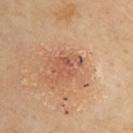Q: Is there a histopathology result?
A: no biopsy performed (imaged during a skin exam)
Q: Patient demographics?
A: male, about 55 years old
Q: What did automated image analysis measure?
A: a lesion–skin lightness drop of about 8 and a lesion-to-skin contrast of about 5.5 (normalized; higher = more distinct)
Q: Illumination type?
A: cross-polarized
Q: Where on the body is the lesion?
A: the upper back
Q: What kind of image is this?
A: ~15 mm crop, total-body skin-cancer survey